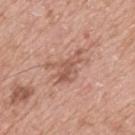workup = no biopsy performed (imaged during a skin exam); subject = male, aged approximately 75; site = the upper back; tile lighting = white-light; imaging modality = ~15 mm crop, total-body skin-cancer survey.